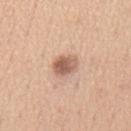Case summary:
* workup · catalogued during a skin exam; not biopsied
* lesion diameter · ~3 mm (longest diameter)
* imaging modality · ~15 mm crop, total-body skin-cancer survey
* body site · the mid back
* subject · male, aged approximately 60